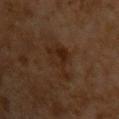biopsy status: catalogued during a skin exam; not biopsied | location: the chest | patient: male, approximately 60 years of age | image source: ~15 mm crop, total-body skin-cancer survey | size: about 4.5 mm.